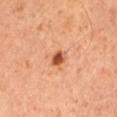Notes:
* biopsy status — total-body-photography surveillance lesion; no biopsy
* automated metrics — a nevus-likeness score of about 100/100 and lesion-presence confidence of about 100/100
* tile lighting — cross-polarized
* acquisition — ~15 mm tile from a whole-body skin photo
* patient — male, roughly 50 years of age
* anatomic site — the left upper arm
* lesion diameter — about 2 mm